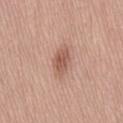lighting: white-light illumination
image-analysis metrics: a lesion area of about 6.5 mm², a shape eccentricity near 0.85, and a symmetry-axis asymmetry near 0.15; a mean CIELAB color near L≈56 a*≈22 b*≈28, about 11 CIELAB-L* units darker than the surrounding skin, and a lesion-to-skin contrast of about 7 (normalized; higher = more distinct)
patient: male, aged around 75
size: ~4 mm (longest diameter)
location: the lower back
acquisition: 15 mm crop, total-body photography